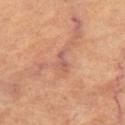Clinical impression:
Imaged during a routine full-body skin examination; the lesion was not biopsied and no histopathology is available.
Acquisition and patient details:
Approximately 2.5 mm at its widest. A 15 mm crop from a total-body photograph taken for skin-cancer surveillance. The lesion is on the left thigh. A subject aged 58 to 62. Imaged with cross-polarized lighting.